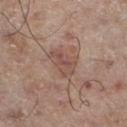Located on the left lower leg.
The patient is a male roughly 70 years of age.
This image is a 15 mm lesion crop taken from a total-body photograph.
This is a white-light tile.